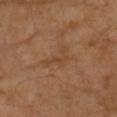{
  "biopsy_status": "not biopsied; imaged during a skin examination",
  "image": {
    "source": "total-body photography crop",
    "field_of_view_mm": 15
  },
  "patient": {
    "sex": "female",
    "age_approx": 70
  },
  "lesion_size": {
    "long_diameter_mm_approx": 4.0
  },
  "automated_metrics": {
    "vs_skin_darker_L": 5.0,
    "border_irregularity_0_10": 7.5,
    "color_variation_0_10": 0.5,
    "peripheral_color_asymmetry": 0.0
  },
  "site": "left forearm"
}